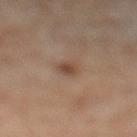<case>
  <lesion_size>
    <long_diameter_mm_approx>2.5</long_diameter_mm_approx>
  </lesion_size>
  <image>
    <source>total-body photography crop</source>
    <field_of_view_mm>15</field_of_view_mm>
  </image>
  <site>left lower leg</site>
  <patient>
    <sex>male</sex>
    <age_approx>65</age_approx>
  </patient>
  <lighting>cross-polarized</lighting>
</case>